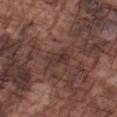Impression:
The lesion was tiled from a total-body skin photograph and was not biopsied.
Clinical summary:
The tile uses white-light illumination. The subject is a male aged 73 to 77. A 15 mm close-up extracted from a 3D total-body photography capture. Approximately 3 mm at its widest. Located on the left forearm.